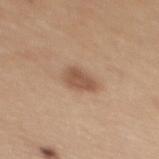Assessment: Part of a total-body skin-imaging series; this lesion was reviewed on a skin check and was not flagged for biopsy. Acquisition and patient details: A 15 mm close-up extracted from a 3D total-body photography capture. Captured under white-light illumination. A female patient roughly 30 years of age. On the upper back.